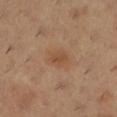This lesion was catalogued during total-body skin photography and was not selected for biopsy.
Cropped from a whole-body photographic skin survey; the tile spans about 15 mm.
This is a cross-polarized tile.
Automated tile analysis of the lesion measured a lesion area of about 4.5 mm² and a shape eccentricity near 0.6. The analysis additionally found an average lesion color of about L≈49 a*≈20 b*≈32 (CIELAB), about 7 CIELAB-L* units darker than the surrounding skin, and a lesion-to-skin contrast of about 5.5 (normalized; higher = more distinct).
The lesion is on the left lower leg.
The lesion's longest dimension is about 2.5 mm.
A female subject aged around 30.